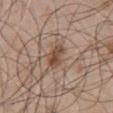The lesion was photographed on a routine skin check and not biopsied; there is no pathology result. On the chest. A male patient in their 50s. Imaged with white-light lighting. Measured at roughly 3 mm in maximum diameter. Automated image analysis of the tile measured a mean CIELAB color near L≈48 a*≈18 b*≈28, a lesion–skin lightness drop of about 10, and a lesion-to-skin contrast of about 8.5 (normalized; higher = more distinct). It also reported a classifier nevus-likeness of about 80/100 and lesion-presence confidence of about 100/100. A close-up tile cropped from a whole-body skin photograph, about 15 mm across.